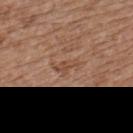On the upper back.
A female patient, aged around 65.
A roughly 15 mm field-of-view crop from a total-body skin photograph.